The lesion is on the arm · a female subject, in their mid-50s · a 15 mm crop from a total-body photograph taken for skin-cancer surveillance.
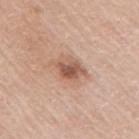{
  "diagnosis": {
    "histopathology": "melanoma in situ",
    "malignancy": "malignant",
    "taxonomic_path": [
      "Malignant",
      "Malignant melanocytic proliferations (Melanoma)",
      "Melanoma in situ"
    ]
  }
}